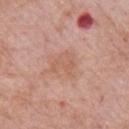Recorded during total-body skin imaging; not selected for excision or biopsy. A female patient, in their 70s. The lesion-visualizer software estimated a lesion area of about 6.5 mm², an outline eccentricity of about 0.5 (0 = round, 1 = elongated), and a symmetry-axis asymmetry near 0.5. It also reported a border-irregularity rating of about 5/10, a within-lesion color-variation index near 2.5/10, and peripheral color asymmetry of about 1. Located on the right upper arm. A 15 mm close-up tile from a total-body photography series done for melanoma screening. The tile uses white-light illumination. About 3 mm across.